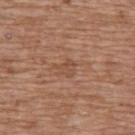* notes — total-body-photography surveillance lesion; no biopsy
* image source — ~15 mm tile from a whole-body skin photo
* tile lighting — white-light illumination
* size — about 2.5 mm
* site — the back
* patient — female, aged 73 to 77